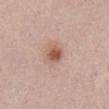Recorded during total-body skin imaging; not selected for excision or biopsy. Imaged with white-light lighting. A female subject roughly 65 years of age. Cropped from a whole-body photographic skin survey; the tile spans about 15 mm. On the abdomen. About 3 mm across.